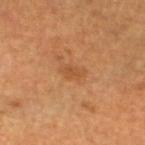Q: Is there a histopathology result?
A: no biopsy performed (imaged during a skin exam)
Q: Who is the patient?
A: female, about 50 years old
Q: How large is the lesion?
A: ~3 mm (longest diameter)
Q: Lesion location?
A: the left lower leg
Q: What kind of image is this?
A: total-body-photography crop, ~15 mm field of view
Q: What lighting was used for the tile?
A: cross-polarized illumination